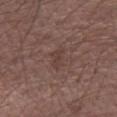notes: no biopsy performed (imaged during a skin exam) | tile lighting: white-light | diameter: ≈2.5 mm | image-analysis metrics: a footprint of about 4 mm² and a symmetry-axis asymmetry near 0.3; a border-irregularity index near 3.5/10, internal color variation of about 2 on a 0–10 scale, and radial color variation of about 0.5; a lesion-detection confidence of about 100/100 | location: the left forearm | imaging modality: total-body-photography crop, ~15 mm field of view | patient: male, about 55 years old.